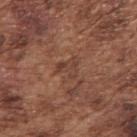Part of a total-body skin-imaging series; this lesion was reviewed on a skin check and was not flagged for biopsy. A male subject aged 73 to 77. The lesion is on the right upper arm. The tile uses white-light illumination. A 15 mm crop from a total-body photograph taken for skin-cancer surveillance.